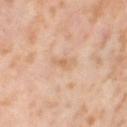notes: total-body-photography surveillance lesion; no biopsy | lighting: cross-polarized illumination | site: the leg | patient: female, aged around 55 | image: ~15 mm crop, total-body skin-cancer survey.